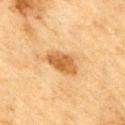The lesion was photographed on a routine skin check and not biopsied; there is no pathology result.
Located on the right upper arm.
A male subject aged approximately 70.
Imaged with cross-polarized lighting.
An algorithmic analysis of the crop reported an area of roughly 8 mm², an eccentricity of roughly 0.8, and a shape-asymmetry score of about 0.2 (0 = symmetric). The analysis additionally found a nevus-likeness score of about 65/100 and a detector confidence of about 100 out of 100 that the crop contains a lesion.
A 15 mm crop from a total-body photograph taken for skin-cancer surveillance.